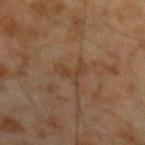Recorded during total-body skin imaging; not selected for excision or biopsy. A male subject, roughly 45 years of age. Longest diameter approximately 3.5 mm. Imaged with cross-polarized lighting. Automated tile analysis of the lesion measured a mean CIELAB color near L≈32 a*≈15 b*≈25 and a normalized border contrast of about 5. The lesion is on the left forearm. A close-up tile cropped from a whole-body skin photograph, about 15 mm across.